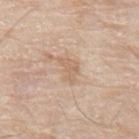Imaged during a routine full-body skin examination; the lesion was not biopsied and no histopathology is available.
The subject is a male aged 78–82.
A region of skin cropped from a whole-body photographic capture, roughly 15 mm wide.
The lesion is on the back.